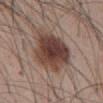Assessment: Captured during whole-body skin photography for melanoma surveillance; the lesion was not biopsied. Image and clinical context: The subject is a male in their mid-40s. A 15 mm crop from a total-body photograph taken for skin-cancer surveillance. The lesion is located on the abdomen. The total-body-photography lesion software estimated a footprint of about 25 mm² and two-axis asymmetry of about 0.2. It also reported a lesion color around L≈42 a*≈18 b*≈23 in CIELAB and roughly 15 lightness units darker than nearby skin. The software also gave a classifier nevus-likeness of about 95/100 and a detector confidence of about 100 out of 100 that the crop contains a lesion. Captured under white-light illumination. Approximately 7 mm at its widest.